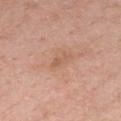tile lighting — white-light
patient — male, in their mid-50s
image — 15 mm crop, total-body photography
anatomic site — the chest
TBP lesion metrics — an area of roughly 2.5 mm², an outline eccentricity of about 0.95 (0 = round, 1 = elongated), and a shape-asymmetry score of about 0.4 (0 = symmetric); roughly 7 lightness units darker than nearby skin and a normalized lesion–skin contrast near 5; border irregularity of about 5 on a 0–10 scale and internal color variation of about 0 on a 0–10 scale; an automated nevus-likeness rating near 0 out of 100
diameter — about 2.5 mm